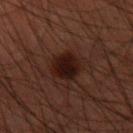Findings:
- workup · catalogued during a skin exam; not biopsied
- image source · 15 mm crop, total-body photography
- size · about 4.5 mm
- illumination · cross-polarized illumination
- subject · male, aged 58 to 62
- anatomic site · the right forearm